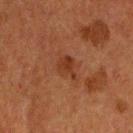biopsy_status: not biopsied; imaged during a skin examination
patient:
  sex: male
  age_approx: 60
lesion_size:
  long_diameter_mm_approx: 2.5
site: head or neck
lighting: cross-polarized
image:
  source: total-body photography crop
  field_of_view_mm: 15